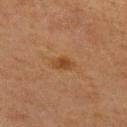| key | value |
|---|---|
| follow-up | catalogued during a skin exam; not biopsied |
| lesion size | ≈2.5 mm |
| automated lesion analysis | an automated nevus-likeness rating near 80 out of 100 |
| subject | female, about 65 years old |
| imaging modality | ~15 mm crop, total-body skin-cancer survey |
| body site | the left upper arm |